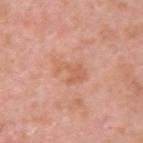workup: total-body-photography surveillance lesion; no biopsy | body site: the back | automated metrics: a footprint of about 7 mm², an eccentricity of roughly 0.8, and a shape-asymmetry score of about 0.4 (0 = symmetric) | tile lighting: white-light | patient: male, roughly 40 years of age | diameter: ~3.5 mm (longest diameter) | acquisition: 15 mm crop, total-body photography.